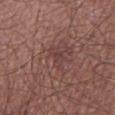No biopsy was performed on this lesion — it was imaged during a full skin examination and was not determined to be concerning. The total-body-photography lesion software estimated a shape eccentricity near 0.8 and two-axis asymmetry of about 0.45. It also reported a lesion color around L≈40 a*≈21 b*≈20 in CIELAB and roughly 6 lightness units darker than nearby skin. It also reported an automated nevus-likeness rating near 0 out of 100. Measured at roughly 3 mm in maximum diameter. A lesion tile, about 15 mm wide, cut from a 3D total-body photograph. From the left lower leg. The subject is a male aged 53 to 57. The tile uses white-light illumination.